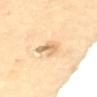Part of a total-body skin-imaging series; this lesion was reviewed on a skin check and was not flagged for biopsy. A female patient, aged 68–72. Located on the upper back. The lesion's longest dimension is about 3 mm. A 15 mm close-up tile from a total-body photography series done for melanoma screening. Imaged with cross-polarized lighting.